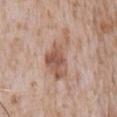Q: Is there a histopathology result?
A: no biopsy performed (imaged during a skin exam)
Q: Where on the body is the lesion?
A: the chest
Q: What kind of image is this?
A: total-body-photography crop, ~15 mm field of view
Q: Patient demographics?
A: male, aged approximately 65
Q: What lighting was used for the tile?
A: white-light illumination
Q: How large is the lesion?
A: ~7 mm (longest diameter)